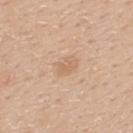Longest diameter approximately 3 mm. A male patient, roughly 30 years of age. Cropped from a whole-body photographic skin survey; the tile spans about 15 mm. From the back. The tile uses white-light illumination.